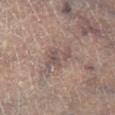This lesion was catalogued during total-body skin photography and was not selected for biopsy. A male subject in their mid- to late 60s. Captured under cross-polarized illumination. On the right lower leg. The total-body-photography lesion software estimated border irregularity of about 5.5 on a 0–10 scale and peripheral color asymmetry of about 1. Cropped from a total-body skin-imaging series; the visible field is about 15 mm. The lesion's longest dimension is about 4 mm.